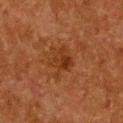On the chest.
A 15 mm crop from a total-body photograph taken for skin-cancer surveillance.
The patient is a female in their 50s.